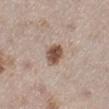Recorded during total-body skin imaging; not selected for excision or biopsy. A female patient about 50 years old. A region of skin cropped from a whole-body photographic capture, roughly 15 mm wide. On the left lower leg.